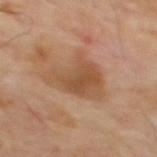notes: catalogued during a skin exam; not biopsied | imaging modality: total-body-photography crop, ~15 mm field of view | lighting: cross-polarized illumination | subject: male, aged 63 to 67 | body site: the upper back | automated metrics: an area of roughly 18 mm², an outline eccentricity of about 0.65 (0 = round, 1 = elongated), and two-axis asymmetry of about 0.45.